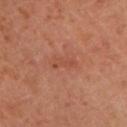The lesion was photographed on a routine skin check and not biopsied; there is no pathology result. Captured under cross-polarized illumination. About 3 mm across. Automated image analysis of the tile measured a footprint of about 3 mm², a shape eccentricity near 0.9, and two-axis asymmetry of about 0.35. The software also gave a lesion color around L≈48 a*≈27 b*≈31 in CIELAB, roughly 6 lightness units darker than nearby skin, and a lesion-to-skin contrast of about 4.5 (normalized; higher = more distinct). Located on the right upper arm. A 15 mm close-up tile from a total-body photography series done for melanoma screening. The patient is a female aged approximately 40.